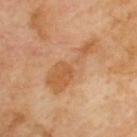follow-up: imaged on a skin check; not biopsied
illumination: cross-polarized illumination
subject: male, aged approximately 70
image source: ~15 mm crop, total-body skin-cancer survey
size: ≈7 mm
anatomic site: the upper back